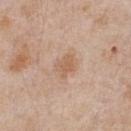Q: Was a biopsy performed?
A: no biopsy performed (imaged during a skin exam)
Q: Where on the body is the lesion?
A: the chest
Q: Patient demographics?
A: male, approximately 65 years of age
Q: What is the lesion's diameter?
A: about 2.5 mm
Q: How was this image acquired?
A: 15 mm crop, total-body photography
Q: What lighting was used for the tile?
A: white-light illumination